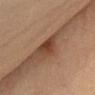biopsy status: imaged on a skin check; not biopsied | site: the front of the torso | size: ≈3 mm | patient: female, aged 63–67 | automated metrics: border irregularity of about 2.5 on a 0–10 scale and radial color variation of about 1 | acquisition: ~15 mm tile from a whole-body skin photo | tile lighting: cross-polarized illumination.